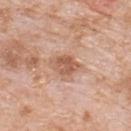Captured during whole-body skin photography for melanoma surveillance; the lesion was not biopsied. From the upper back. Measured at roughly 3.5 mm in maximum diameter. A male patient roughly 80 years of age. The lesion-visualizer software estimated an average lesion color of about L≈58 a*≈22 b*≈31 (CIELAB) and a normalized border contrast of about 7.5. The tile uses white-light illumination. Cropped from a whole-body photographic skin survey; the tile spans about 15 mm.